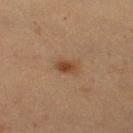follow-up: total-body-photography surveillance lesion; no biopsy | automated metrics: a border-irregularity rating of about 2/10 and a peripheral color-asymmetry measure near 1 | patient: female, in their 40s | diameter: about 2.5 mm | imaging modality: ~15 mm crop, total-body skin-cancer survey | anatomic site: the leg.